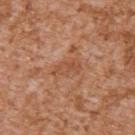  biopsy_status: not biopsied; imaged during a skin examination
  site: arm
  image:
    source: total-body photography crop
    field_of_view_mm: 15
  patient:
    sex: male
    age_approx: 45
  lighting: white-light
  lesion_size:
    long_diameter_mm_approx: 3.5